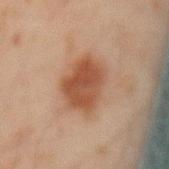Clinical impression:
Imaged during a routine full-body skin examination; the lesion was not biopsied and no histopathology is available.
Acquisition and patient details:
The lesion-visualizer software estimated a border-irregularity index near 2.5/10 and a within-lesion color-variation index near 3/10. The lesion is on the lower back. A close-up tile cropped from a whole-body skin photograph, about 15 mm across. Imaged with cross-polarized lighting. A male patient, roughly 45 years of age. Longest diameter approximately 5.5 mm.